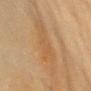Assessment:
The lesion was tiled from a total-body skin photograph and was not biopsied.
Clinical summary:
A roughly 15 mm field-of-view crop from a total-body skin photograph. A female patient, aged 58 to 62. The tile uses cross-polarized illumination. About 8.5 mm across. On the front of the torso. The lesion-visualizer software estimated an area of roughly 15 mm², a shape eccentricity near 0.95, and two-axis asymmetry of about 0.3. The software also gave a mean CIELAB color near L≈52 a*≈17 b*≈35, roughly 6 lightness units darker than nearby skin, and a normalized lesion–skin contrast near 5. The software also gave a border-irregularity rating of about 6/10.